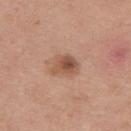workup: no biopsy performed (imaged during a skin exam)
imaging modality: 15 mm crop, total-body photography
illumination: white-light illumination
site: the upper back
subject: male, approximately 25 years of age
TBP lesion metrics: border irregularity of about 3.5 on a 0–10 scale; a nevus-likeness score of about 80/100 and a detector confidence of about 100 out of 100 that the crop contains a lesion